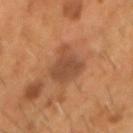subject = male, in their mid-50s; imaging modality = 15 mm crop, total-body photography; lighting = cross-polarized illumination; location = the head or neck.